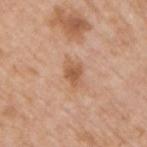This lesion was catalogued during total-body skin photography and was not selected for biopsy.